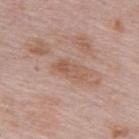The lesion was tiled from a total-body skin photograph and was not biopsied. The lesion is on the upper back. Approximately 3.5 mm at its widest. The patient is a female aged 63–67. This image is a 15 mm lesion crop taken from a total-body photograph. This is a white-light tile.